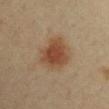<tbp_lesion>
<biopsy_status>not biopsied; imaged during a skin examination</biopsy_status>
<image>
  <source>total-body photography crop</source>
  <field_of_view_mm>15</field_of_view_mm>
</image>
<lesion_size>
  <long_diameter_mm_approx>5.0</long_diameter_mm_approx>
</lesion_size>
<site>arm</site>
<patient>
  <sex>male</sex>
  <age_approx>40</age_approx>
</patient>
</tbp_lesion>